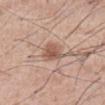Imaged during a routine full-body skin examination; the lesion was not biopsied and no histopathology is available. A close-up tile cropped from a whole-body skin photograph, about 15 mm across. The lesion-visualizer software estimated a shape eccentricity near 0.55 and two-axis asymmetry of about 0.2. It also reported border irregularity of about 1.5 on a 0–10 scale and peripheral color asymmetry of about 1.5. About 3.5 mm across. Located on the abdomen. This is a white-light tile. The patient is a male aged around 55.